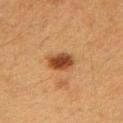TBP lesion metrics = a border-irregularity index near 2/10
tile lighting = cross-polarized
diameter = ≈3.5 mm
image source = 15 mm crop, total-body photography
anatomic site = the right upper arm
patient = female, approximately 40 years of age